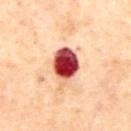Notes:
– site — the abdomen
– image source — ~15 mm tile from a whole-body skin photo
– subject — male, aged 68 to 72